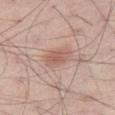Notes:
– biopsy status · no biopsy performed (imaged during a skin exam)
– body site · the left thigh
– acquisition · ~15 mm crop, total-body skin-cancer survey
– diameter · ~3.5 mm (longest diameter)
– subject · male, aged approximately 55